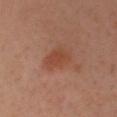| field | value |
|---|---|
| biopsy status | imaged on a skin check; not biopsied |
| location | the head or neck |
| patient | female, aged around 30 |
| image source | ~15 mm crop, total-body skin-cancer survey |
| lesion size | about 3.5 mm |
| illumination | cross-polarized illumination |
| automated lesion analysis | a lesion area of about 8 mm², a shape eccentricity near 0.55, and a symmetry-axis asymmetry near 0.3; a classifier nevus-likeness of about 50/100 and lesion-presence confidence of about 100/100 |